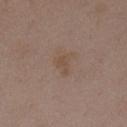The lesion was photographed on a routine skin check and not biopsied; there is no pathology result. A female subject aged around 35. Imaged with white-light lighting. The lesion is on the front of the torso. The recorded lesion diameter is about 2.5 mm. Automated image analysis of the tile measured a footprint of about 4 mm², an outline eccentricity of about 0.7 (0 = round, 1 = elongated), and a shape-asymmetry score of about 0.45 (0 = symmetric). Cropped from a total-body skin-imaging series; the visible field is about 15 mm.